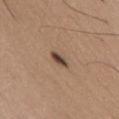An algorithmic analysis of the crop reported an outline eccentricity of about 0.9 (0 = round, 1 = elongated) and two-axis asymmetry of about 0.25. The analysis additionally found a mean CIELAB color near L≈45 a*≈17 b*≈26 and a lesion-to-skin contrast of about 10.5 (normalized; higher = more distinct). The software also gave an automated nevus-likeness rating near 0 out of 100 and lesion-presence confidence of about 100/100. Located on the abdomen. Approximately 3 mm at its widest. A male patient, aged 28–32. A region of skin cropped from a whole-body photographic capture, roughly 15 mm wide.